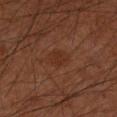The lesion was tiled from a total-body skin photograph and was not biopsied.
Approximately 3 mm at its widest.
Located on the right forearm.
A male subject, in their 60s.
Cropped from a whole-body photographic skin survey; the tile spans about 15 mm.
An algorithmic analysis of the crop reported a lesion color around L≈27 a*≈20 b*≈26 in CIELAB, about 4 CIELAB-L* units darker than the surrounding skin, and a lesion-to-skin contrast of about 5 (normalized; higher = more distinct). The analysis additionally found a border-irregularity rating of about 2/10 and a peripheral color-asymmetry measure near 0.5.
Captured under cross-polarized illumination.